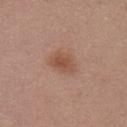- follow-up: catalogued during a skin exam; not biopsied
- lesion diameter: ~3.5 mm (longest diameter)
- subject: female, aged 33–37
- location: the chest
- imaging modality: ~15 mm tile from a whole-body skin photo